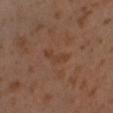workup=catalogued during a skin exam; not biopsied | body site=the leg | patient=male, roughly 30 years of age | image=total-body-photography crop, ~15 mm field of view | lighting=cross-polarized illumination.